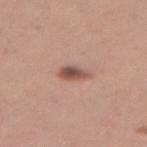Case summary:
• follow-up — no biopsy performed (imaged during a skin exam)
• image source — 15 mm crop, total-body photography
• location — the leg
• patient — female, aged around 30
• lighting — white-light
• TBP lesion metrics — an area of roughly 4.5 mm²; an average lesion color of about L≈52 a*≈21 b*≈25 (CIELAB), a lesion–skin lightness drop of about 13, and a normalized lesion–skin contrast near 8.5; internal color variation of about 5 on a 0–10 scale and radial color variation of about 1.5
• lesion diameter — ≈3 mm